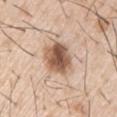* notes · no biopsy performed (imaged during a skin exam)
* acquisition · 15 mm crop, total-body photography
* size · ~4.5 mm (longest diameter)
* patient · male, aged around 55
* illumination · white-light illumination